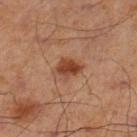Background:
The patient is a male aged 68–72. Imaged with cross-polarized lighting. This image is a 15 mm lesion crop taken from a total-body photograph. An algorithmic analysis of the crop reported an area of roughly 4.5 mm² and a symmetry-axis asymmetry near 0.25. It also reported a lesion–skin lightness drop of about 11 and a normalized lesion–skin contrast near 10. The analysis additionally found border irregularity of about 2 on a 0–10 scale, a color-variation rating of about 2.5/10, and a peripheral color-asymmetry measure near 1. The software also gave an automated nevus-likeness rating near 95 out of 100 and a lesion-detection confidence of about 100/100. Located on the left lower leg. Approximately 3 mm at its widest.